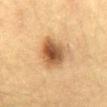notes: no biopsy performed (imaged during a skin exam)
acquisition: total-body-photography crop, ~15 mm field of view
patient: female, aged approximately 30
location: the abdomen